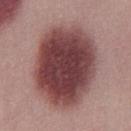{"biopsy_status": "not biopsied; imaged during a skin examination", "automated_metrics": {"eccentricity": 0.55, "shape_asymmetry": 0.1, "cielab_L": 42, "cielab_a": 24, "cielab_b": 19, "nevus_likeness_0_100": 95, "lesion_detection_confidence_0_100": 100}, "lighting": "white-light", "patient": {"sex": "male", "age_approx": 30}, "image": {"source": "total-body photography crop", "field_of_view_mm": 15}, "lesion_size": {"long_diameter_mm_approx": 9.5}}